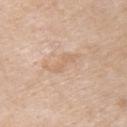| feature | finding |
|---|---|
| notes | catalogued during a skin exam; not biopsied |
| diameter | ≈4 mm |
| tile lighting | white-light illumination |
| site | the upper back |
| subject | male, aged approximately 85 or older |
| imaging modality | ~15 mm crop, total-body skin-cancer survey |